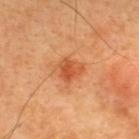notes: total-body-photography surveillance lesion; no biopsy | tile lighting: cross-polarized illumination | lesion size: about 2.5 mm | imaging modality: total-body-photography crop, ~15 mm field of view | automated lesion analysis: a border-irregularity rating of about 3/10, a color-variation rating of about 3.5/10, and peripheral color asymmetry of about 1 | site: the upper back | patient: male, aged 43 to 47.